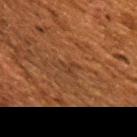{
  "biopsy_status": "not biopsied; imaged during a skin examination",
  "site": "upper back",
  "lesion_size": {
    "long_diameter_mm_approx": 3.5
  },
  "patient": {
    "sex": "female",
    "age_approx": 50
  },
  "image": {
    "source": "total-body photography crop",
    "field_of_view_mm": 15
  },
  "automated_metrics": {
    "cielab_L": 31,
    "cielab_a": 19,
    "cielab_b": 28,
    "vs_skin_darker_L": 5.0,
    "vs_skin_contrast_norm": 5.0,
    "border_irregularity_0_10": 6.0,
    "color_variation_0_10": 0.0,
    "peripheral_color_asymmetry": 0.0,
    "nevus_likeness_0_100": 0,
    "lesion_detection_confidence_0_100": 60
  }
}